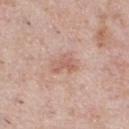workup — no biopsy performed (imaged during a skin exam) | location — the chest | lesion diameter — ~3 mm (longest diameter) | imaging modality — 15 mm crop, total-body photography | patient — male, roughly 40 years of age | TBP lesion metrics — a shape eccentricity near 0.7; a mean CIELAB color near L≈61 a*≈21 b*≈27, roughly 8 lightness units darker than nearby skin, and a lesion-to-skin contrast of about 5.5 (normalized; higher = more distinct).